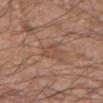follow-up = catalogued during a skin exam; not biopsied
lesion diameter = about 3 mm
body site = the left upper arm
image = ~15 mm tile from a whole-body skin photo
patient = male, roughly 65 years of age